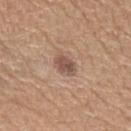Impression: The lesion was tiled from a total-body skin photograph and was not biopsied. Image and clinical context: An algorithmic analysis of the crop reported a border-irregularity rating of about 2/10 and internal color variation of about 2.5 on a 0–10 scale. The lesion is located on the left upper arm. The tile uses white-light illumination. A male patient, about 60 years old. Measured at roughly 3 mm in maximum diameter. A roughly 15 mm field-of-view crop from a total-body skin photograph.